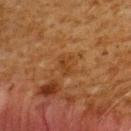Imaged during a routine full-body skin examination; the lesion was not biopsied and no histopathology is available. Imaged with cross-polarized lighting. The recorded lesion diameter is about 2.5 mm. On the upper back. This image is a 15 mm lesion crop taken from a total-body photograph. A male subject, approximately 60 years of age. Automated image analysis of the tile measured an outline eccentricity of about 0.75 (0 = round, 1 = elongated) and a shape-asymmetry score of about 0.35 (0 = symmetric). The software also gave a mean CIELAB color near L≈34 a*≈20 b*≈33 and a normalized lesion–skin contrast near 5.5. The analysis additionally found radial color variation of about 0.5.